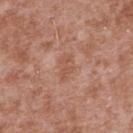{
  "biopsy_status": "not biopsied; imaged during a skin examination",
  "lighting": "white-light",
  "site": "upper back",
  "image": {
    "source": "total-body photography crop",
    "field_of_view_mm": 15
  },
  "lesion_size": {
    "long_diameter_mm_approx": 3.5
  },
  "patient": {
    "sex": "male",
    "age_approx": 45
  },
  "automated_metrics": {
    "shape_asymmetry": 0.25,
    "cielab_L": 54,
    "cielab_a": 23,
    "cielab_b": 30,
    "vs_skin_darker_L": 6.0,
    "vs_skin_contrast_norm": 5.0,
    "lesion_detection_confidence_0_100": 100
  }
}